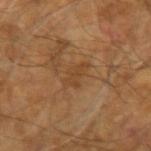Assessment: The lesion was photographed on a routine skin check and not biopsied; there is no pathology result. Image and clinical context: Captured under cross-polarized illumination. A male subject approximately 60 years of age. The lesion is on the left arm. Longest diameter approximately 3.5 mm. An algorithmic analysis of the crop reported two-axis asymmetry of about 0.4. This image is a 15 mm lesion crop taken from a total-body photograph.